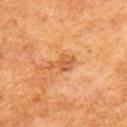Q: Was a biopsy performed?
A: total-body-photography surveillance lesion; no biopsy
Q: How large is the lesion?
A: ~3.5 mm (longest diameter)
Q: What did automated image analysis measure?
A: border irregularity of about 4.5 on a 0–10 scale and peripheral color asymmetry of about 1
Q: Lesion location?
A: the back
Q: What are the patient's age and sex?
A: male, aged 58–62
Q: How was this image acquired?
A: total-body-photography crop, ~15 mm field of view
Q: Illumination type?
A: cross-polarized